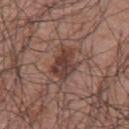workup: total-body-photography surveillance lesion; no biopsy | site: the left arm | patient: male, aged approximately 70 | automated metrics: a mean CIELAB color near L≈39 a*≈20 b*≈23, roughly 11 lightness units darker than nearby skin, and a lesion-to-skin contrast of about 9 (normalized; higher = more distinct); a border-irregularity rating of about 3/10, internal color variation of about 4.5 on a 0–10 scale, and a peripheral color-asymmetry measure near 1.5 | tile lighting: white-light | image: total-body-photography crop, ~15 mm field of view | size: ~3.5 mm (longest diameter).